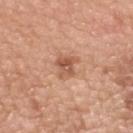follow-up: no biopsy performed (imaged during a skin exam)
lighting: white-light
anatomic site: the upper back
image source: ~15 mm tile from a whole-body skin photo
image-analysis metrics: a lesion area of about 5.5 mm², a shape eccentricity near 0.6, and a shape-asymmetry score of about 0.35 (0 = symmetric); a border-irregularity index near 4/10 and internal color variation of about 5.5 on a 0–10 scale; a nevus-likeness score of about 35/100
size: about 3 mm
subject: male, aged 48–52